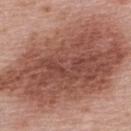• follow-up · imaged on a skin check; not biopsied
• tile lighting · white-light illumination
• patient · female, aged 48 to 52
• imaging modality · ~15 mm crop, total-body skin-cancer survey
• site · the upper back
• diameter · about 17 mm